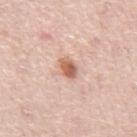Clinical impression:
Captured during whole-body skin photography for melanoma surveillance; the lesion was not biopsied.
Context:
A roughly 15 mm field-of-view crop from a total-body skin photograph. An algorithmic analysis of the crop reported a shape eccentricity near 0.8 and a symmetry-axis asymmetry near 0.15. It also reported a mean CIELAB color near L≈61 a*≈24 b*≈30, about 14 CIELAB-L* units darker than the surrounding skin, and a normalized lesion–skin contrast near 9. The software also gave border irregularity of about 1.5 on a 0–10 scale and a color-variation rating of about 4/10. It also reported a nevus-likeness score of about 95/100. Located on the abdomen. Approximately 2.5 mm at its widest. A male subject aged 73–77.